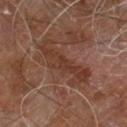No biopsy was performed on this lesion — it was imaged during a full skin examination and was not determined to be concerning. The lesion is on the right leg. Automated image analysis of the tile measured a footprint of about 14 mm² and a shape eccentricity near 0.9. It also reported a lesion-detection confidence of about 100/100. About 6.5 mm across. The subject is a male aged 58 to 62. Cropped from a whole-body photographic skin survey; the tile spans about 15 mm.